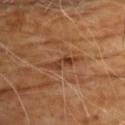Assessment:
Imaged during a routine full-body skin examination; the lesion was not biopsied and no histopathology is available.
Context:
The recorded lesion diameter is about 4 mm. A male patient, approximately 70 years of age. From the chest. A 15 mm close-up extracted from a 3D total-body photography capture. The tile uses cross-polarized illumination.